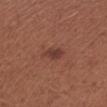Impression: Captured during whole-body skin photography for melanoma surveillance; the lesion was not biopsied. Image and clinical context: On the right upper arm. A close-up tile cropped from a whole-body skin photograph, about 15 mm across. Automated tile analysis of the lesion measured a shape eccentricity near 0.75. And it measured a lesion color around L≈38 a*≈23 b*≈25 in CIELAB. The software also gave a border-irregularity index near 2.5/10, internal color variation of about 1.5 on a 0–10 scale, and peripheral color asymmetry of about 0.5. The software also gave a nevus-likeness score of about 50/100 and lesion-presence confidence of about 100/100. The subject is a female in their mid- to late 50s. The lesion's longest dimension is about 2.5 mm.